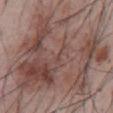follow-up: catalogued during a skin exam; not biopsied.